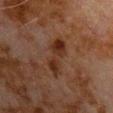A close-up tile cropped from a whole-body skin photograph, about 15 mm across.
The subject is a male aged 78–82.
Measured at roughly 5 mm in maximum diameter.
Captured under cross-polarized illumination.
On the chest.